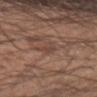No biopsy was performed on this lesion — it was imaged during a full skin examination and was not determined to be concerning. The patient is a male approximately 35 years of age. About 3 mm across. A 15 mm close-up extracted from a 3D total-body photography capture. The lesion is located on the arm. This is a white-light tile.